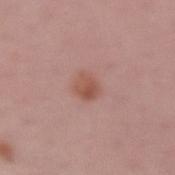Imaged during a routine full-body skin examination; the lesion was not biopsied and no histopathology is available.
Imaged with white-light lighting.
The lesion is on the left forearm.
An algorithmic analysis of the crop reported an automated nevus-likeness rating near 75 out of 100 and a lesion-detection confidence of about 100/100.
The subject is a female aged 43–47.
The lesion's longest dimension is about 2.5 mm.
Cropped from a whole-body photographic skin survey; the tile spans about 15 mm.